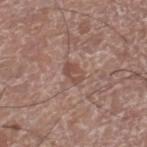The lesion was photographed on a routine skin check and not biopsied; there is no pathology result. Captured under white-light illumination. Longest diameter approximately 2.5 mm. A male patient in their mid-70s. Cropped from a whole-body photographic skin survey; the tile spans about 15 mm. The total-body-photography lesion software estimated about 8 CIELAB-L* units darker than the surrounding skin and a normalized lesion–skin contrast near 6. The analysis additionally found a border-irregularity index near 2/10 and peripheral color asymmetry of about 1.5. Located on the right lower leg.